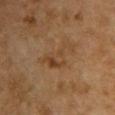This lesion was catalogued during total-body skin photography and was not selected for biopsy. The patient is a female about 60 years old. This image is a 15 mm lesion crop taken from a total-body photograph. On the front of the torso.